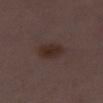workup — no biopsy performed (imaged during a skin exam) | lighting — white-light illumination | anatomic site — the left thigh | lesion size — ≈3.5 mm | acquisition — ~15 mm tile from a whole-body skin photo | subject — female, aged around 30.